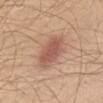Case summary:
• follow-up · imaged on a skin check; not biopsied
• subject · male, roughly 60 years of age
• image · ~15 mm crop, total-body skin-cancer survey
• anatomic site · the front of the torso
• lesion diameter · ~4.5 mm (longest diameter)
• automated metrics · an average lesion color of about L≈55 a*≈23 b*≈29 (CIELAB) and a lesion–skin lightness drop of about 11; a color-variation rating of about 2.5/10 and a peripheral color-asymmetry measure near 1